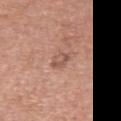Recorded during total-body skin imaging; not selected for excision or biopsy. Measured at roughly 2.5 mm in maximum diameter. The patient is a female aged approximately 60. Imaged with white-light lighting. From the back. A 15 mm close-up tile from a total-body photography series done for melanoma screening.